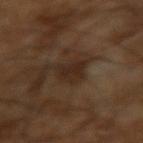Background: Automated tile analysis of the lesion measured an average lesion color of about L≈24 a*≈14 b*≈23 (CIELAB), roughly 7 lightness units darker than nearby skin, and a normalized border contrast of about 7.5. The software also gave a border-irregularity rating of about 4/10, a within-lesion color-variation index near 3/10, and peripheral color asymmetry of about 1. On the right arm. Cropped from a whole-body photographic skin survey; the tile spans about 15 mm. Approximately 3 mm at its widest. A male patient, about 60 years old. Captured under cross-polarized illumination.